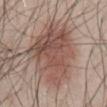The lesion was photographed on a routine skin check and not biopsied; there is no pathology result.
The total-body-photography lesion software estimated a border-irregularity rating of about 5.5/10, internal color variation of about 5.5 on a 0–10 scale, and a peripheral color-asymmetry measure near 2. And it measured a classifier nevus-likeness of about 75/100 and a lesion-detection confidence of about 100/100.
The lesion is on the abdomen.
The patient is a male approximately 50 years of age.
A 15 mm close-up extracted from a 3D total-body photography capture.